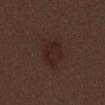notes: catalogued during a skin exam; not biopsied
image source: total-body-photography crop, ~15 mm field of view
site: the chest
patient: female, approximately 30 years of age
illumination: white-light illumination